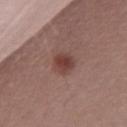{"biopsy_status": "not biopsied; imaged during a skin examination", "image": {"source": "total-body photography crop", "field_of_view_mm": 15}, "lesion_size": {"long_diameter_mm_approx": 3.5}, "automated_metrics": {"shape_asymmetry": 0.15, "cielab_L": 42, "cielab_a": 21, "cielab_b": 23, "vs_skin_darker_L": 10.0, "vs_skin_contrast_norm": 8.0, "nevus_likeness_0_100": 95, "lesion_detection_confidence_0_100": 100}, "patient": {"sex": "female", "age_approx": 40}, "site": "front of the torso", "lighting": "white-light"}